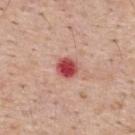notes: imaged on a skin check; not biopsied | site: the back | image: ~15 mm crop, total-body skin-cancer survey | tile lighting: white-light illumination | subject: male, about 55 years old | diameter: about 2.5 mm.